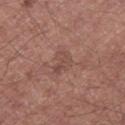Q: Was this lesion biopsied?
A: total-body-photography surveillance lesion; no biopsy
Q: Patient demographics?
A: male, aged approximately 55
Q: What is the anatomic site?
A: the left lower leg
Q: How large is the lesion?
A: ≈3 mm
Q: Automated lesion metrics?
A: an outline eccentricity of about 0.75 (0 = round, 1 = elongated) and a symmetry-axis asymmetry near 0.45; an average lesion color of about L≈47 a*≈20 b*≈24 (CIELAB), about 6 CIELAB-L* units darker than the surrounding skin, and a normalized border contrast of about 5
Q: How was this image acquired?
A: 15 mm crop, total-body photography
Q: Illumination type?
A: white-light